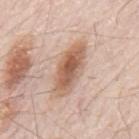This lesion was catalogued during total-body skin photography and was not selected for biopsy. A close-up tile cropped from a whole-body skin photograph, about 15 mm across. On the mid back. The recorded lesion diameter is about 6.5 mm. The lesion-visualizer software estimated a footprint of about 14 mm², a shape eccentricity near 0.9, and two-axis asymmetry of about 0.15. The software also gave roughly 13 lightness units darker than nearby skin and a lesion-to-skin contrast of about 9 (normalized; higher = more distinct). The analysis additionally found a classifier nevus-likeness of about 100/100. A male patient aged around 80.